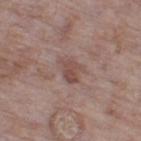Image and clinical context: Approximately 3 mm at its widest. The lesion-visualizer software estimated an outline eccentricity of about 0.7 (0 = round, 1 = elongated) and a symmetry-axis asymmetry near 0.35. And it measured a mean CIELAB color near L≈48 a*≈19 b*≈22, roughly 8 lightness units darker than nearby skin, and a normalized lesion–skin contrast near 6.5. The subject is a female about 85 years old or older. This image is a 15 mm lesion crop taken from a total-body photograph. On the left thigh.